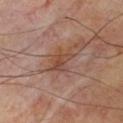| feature | finding |
|---|---|
| workup | imaged on a skin check; not biopsied |